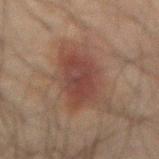The recorded lesion diameter is about 6 mm. Captured under cross-polarized illumination. A male patient, aged 58 to 62. A roughly 15 mm field-of-view crop from a total-body skin photograph. Located on the abdomen. Automated tile analysis of the lesion measured a border-irregularity index near 3/10 and radial color variation of about 1. The analysis additionally found a classifier nevus-likeness of about 95/100 and lesion-presence confidence of about 100/100.